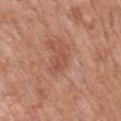The lesion is on the back. A male patient, aged approximately 70. Automated tile analysis of the lesion measured a mean CIELAB color near L≈51 a*≈24 b*≈30, about 7 CIELAB-L* units darker than the surrounding skin, and a lesion-to-skin contrast of about 5 (normalized; higher = more distinct). The analysis additionally found a border-irregularity index near 3.5/10, a within-lesion color-variation index near 1.5/10, and peripheral color asymmetry of about 0.5. A 15 mm close-up extracted from a 3D total-body photography capture.